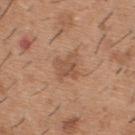Notes:
• notes — imaged on a skin check; not biopsied
• illumination — white-light
• image — ~15 mm crop, total-body skin-cancer survey
• image-analysis metrics — an average lesion color of about L≈53 a*≈20 b*≈32 (CIELAB) and about 8 CIELAB-L* units darker than the surrounding skin; an automated nevus-likeness rating near 10 out of 100 and a detector confidence of about 100 out of 100 that the crop contains a lesion
• subject — male, approximately 40 years of age
• location — the upper back
• size — about 3.5 mm